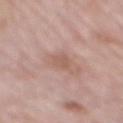• notes — catalogued during a skin exam; not biopsied
• lesion size — about 2.5 mm
• patient — male, in their mid-70s
• imaging modality — 15 mm crop, total-body photography
• location — the mid back